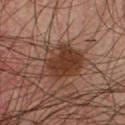{
  "biopsy_status": "not biopsied; imaged during a skin examination",
  "site": "chest",
  "automated_metrics": {
    "area_mm2_approx": 17.0,
    "shape_asymmetry": 0.2,
    "cielab_L": 36,
    "cielab_a": 20,
    "cielab_b": 27,
    "vs_skin_darker_L": 11.0,
    "vs_skin_contrast_norm": 9.5,
    "nevus_likeness_0_100": 95,
    "lesion_detection_confidence_0_100": 100
  },
  "patient": {
    "sex": "male",
    "age_approx": 35
  },
  "lesion_size": {
    "long_diameter_mm_approx": 6.0
  },
  "image": {
    "source": "total-body photography crop",
    "field_of_view_mm": 15
  },
  "lighting": "cross-polarized"
}